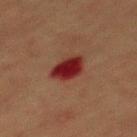Captured during whole-body skin photography for melanoma surveillance; the lesion was not biopsied.
Longest diameter approximately 3.5 mm.
This is a cross-polarized tile.
The lesion is on the mid back.
The subject is a male aged around 40.
A close-up tile cropped from a whole-body skin photograph, about 15 mm across.